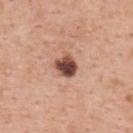workup: total-body-photography surveillance lesion; no biopsy | illumination: white-light illumination | acquisition: ~15 mm crop, total-body skin-cancer survey | patient: female, roughly 50 years of age | diameter: ≈3 mm | automated metrics: a footprint of about 6 mm², an outline eccentricity of about 0.45 (0 = round, 1 = elongated), and two-axis asymmetry of about 0.2; a border-irregularity rating of about 2/10, internal color variation of about 7.5 on a 0–10 scale, and peripheral color asymmetry of about 2.5 | body site: the upper back.